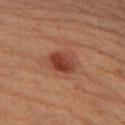Notes:
- workup — catalogued during a skin exam; not biopsied
- patient — female, aged 38–42
- imaging modality — 15 mm crop, total-body photography
- location — the left lower leg